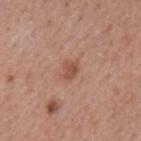Background: Cropped from a total-body skin-imaging series; the visible field is about 15 mm. Longest diameter approximately 2.5 mm. The subject is a male aged around 65. Imaged with white-light lighting. Located on the mid back.